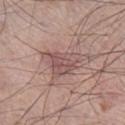{"site": "right lower leg", "patient": {"sex": "male", "age_approx": 70}, "lesion_size": {"long_diameter_mm_approx": 5.5}, "image": {"source": "total-body photography crop", "field_of_view_mm": 15}, "lighting": "white-light"}